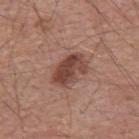Recorded during total-body skin imaging; not selected for excision or biopsy. From the mid back. A roughly 15 mm field-of-view crop from a total-body skin photograph. A male subject in their mid- to late 50s.